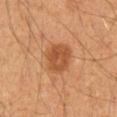Impression: The lesion was photographed on a routine skin check and not biopsied; there is no pathology result. Clinical summary: The lesion is located on the mid back. The tile uses cross-polarized illumination. The recorded lesion diameter is about 3.5 mm. A male patient aged around 60. Cropped from a whole-body photographic skin survey; the tile spans about 15 mm.